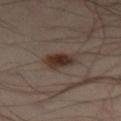Impression:
No biopsy was performed on this lesion — it was imaged during a full skin examination and was not determined to be concerning.